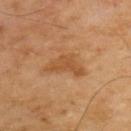Part of a total-body skin-imaging series; this lesion was reviewed on a skin check and was not flagged for biopsy. A male patient, aged approximately 55. A 15 mm crop from a total-body photograph taken for skin-cancer surveillance. The lesion-visualizer software estimated a lesion area of about 8 mm² and a symmetry-axis asymmetry near 0.55. The software also gave an average lesion color of about L≈48 a*≈21 b*≈37 (CIELAB), a lesion–skin lightness drop of about 8, and a lesion-to-skin contrast of about 6.5 (normalized; higher = more distinct). It also reported a border-irregularity index near 6/10 and a color-variation rating of about 1.5/10. The software also gave a lesion-detection confidence of about 100/100. Captured under cross-polarized illumination. The lesion is on the back. The recorded lesion diameter is about 5 mm.